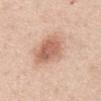Assessment: No biopsy was performed on this lesion — it was imaged during a full skin examination and was not determined to be concerning. Clinical summary: A male patient aged 48–52. About 6.5 mm across. Cropped from a total-body skin-imaging series; the visible field is about 15 mm. Imaged with white-light lighting. The lesion is on the abdomen.